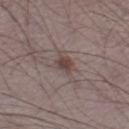No biopsy was performed on this lesion — it was imaged during a full skin examination and was not determined to be concerning.